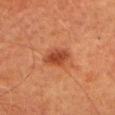notes = imaged on a skin check; not biopsied | subject = female, aged around 60 | imaging modality = ~15 mm crop, total-body skin-cancer survey | size = ~3.5 mm (longest diameter) | illumination = cross-polarized illumination | site = the head or neck.